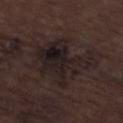{
  "biopsy_status": "not biopsied; imaged during a skin examination",
  "automated_metrics": {
    "area_mm2_approx": 25.0,
    "eccentricity": 0.65,
    "shape_asymmetry": 0.45,
    "cielab_L": 19,
    "cielab_a": 10,
    "cielab_b": 12,
    "vs_skin_darker_L": 6.0,
    "vs_skin_contrast_norm": 9.5
  },
  "patient": {
    "sex": "male",
    "age_approx": 70
  },
  "lesion_size": {
    "long_diameter_mm_approx": 7.5
  },
  "lighting": "white-light",
  "image": {
    "source": "total-body photography crop",
    "field_of_view_mm": 15
  },
  "site": "left lower leg"
}